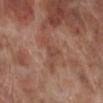site — the right lower leg | illumination — white-light illumination | diameter — ≈4 mm | subject — male, about 70 years old | imaging modality — 15 mm crop, total-body photography.